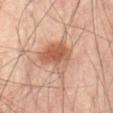No biopsy was performed on this lesion — it was imaged during a full skin examination and was not determined to be concerning. From the abdomen. A male patient approximately 65 years of age. Imaged with cross-polarized lighting. A 15 mm close-up tile from a total-body photography series done for melanoma screening. An algorithmic analysis of the crop reported a lesion-to-skin contrast of about 8 (normalized; higher = more distinct). The lesion's longest dimension is about 4.5 mm.